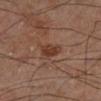Case summary:
• acquisition — total-body-photography crop, ~15 mm field of view
• lesion size — about 3 mm
• subject — male, in their mid-60s
• location — the right lower leg
• automated metrics — a mean CIELAB color near L≈30 a*≈18 b*≈24, about 8 CIELAB-L* units darker than the surrounding skin, and a normalized lesion–skin contrast near 8; border irregularity of about 2 on a 0–10 scale